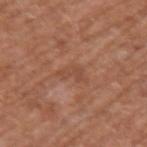{
  "biopsy_status": "not biopsied; imaged during a skin examination",
  "site": "upper back",
  "automated_metrics": {
    "cielab_L": 48,
    "cielab_a": 23,
    "cielab_b": 31,
    "vs_skin_darker_L": 6.0,
    "vs_skin_contrast_norm": 4.5,
    "peripheral_color_asymmetry": 0.0,
    "nevus_likeness_0_100": 0,
    "lesion_detection_confidence_0_100": 100
  },
  "image": {
    "source": "total-body photography crop",
    "field_of_view_mm": 15
  },
  "patient": {
    "sex": "male",
    "age_approx": 65
  }
}